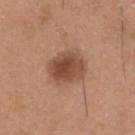Impression: Imaged during a routine full-body skin examination; the lesion was not biopsied and no histopathology is available. Clinical summary: Located on the head or neck. The tile uses white-light illumination. About 4 mm across. Cropped from a total-body skin-imaging series; the visible field is about 15 mm. A male subject, aged 43 to 47. Automated tile analysis of the lesion measured an area of roughly 13 mm², a shape eccentricity near 0.55, and two-axis asymmetry of about 0.15.